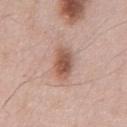Impression:
Imaged during a routine full-body skin examination; the lesion was not biopsied and no histopathology is available.
Context:
The tile uses white-light illumination. From the chest. About 4 mm across. A male patient in their mid-50s. This image is a 15 mm lesion crop taken from a total-body photograph.